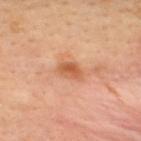Imaged during a routine full-body skin examination; the lesion was not biopsied and no histopathology is available.
Automated tile analysis of the lesion measured roughly 10 lightness units darker than nearby skin and a normalized lesion–skin contrast near 7.5. The software also gave a border-irregularity rating of about 1.5/10, a within-lesion color-variation index near 4.5/10, and a peripheral color-asymmetry measure near 1.5. The software also gave a classifier nevus-likeness of about 35/100 and a detector confidence of about 100 out of 100 that the crop contains a lesion.
Captured under cross-polarized illumination.
On the back.
Cropped from a total-body skin-imaging series; the visible field is about 15 mm.
The lesion's longest dimension is about 3 mm.
The subject is a male in their mid- to late 30s.